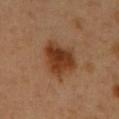anatomic site = the chest
patient = male, aged 48–52
imaging modality = 15 mm crop, total-body photography
tile lighting = cross-polarized illumination